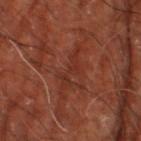No biopsy was performed on this lesion — it was imaged during a full skin examination and was not determined to be concerning. Automated image analysis of the tile measured a within-lesion color-variation index near 0/10. A close-up tile cropped from a whole-body skin photograph, about 15 mm across. Imaged with cross-polarized lighting. From the right thigh. Longest diameter approximately 5 mm. The subject is aged around 65.